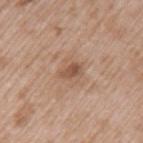Clinical impression: Recorded during total-body skin imaging; not selected for excision or biopsy. Context: A male patient, aged 48 to 52. The recorded lesion diameter is about 2.5 mm. Cropped from a whole-body photographic skin survey; the tile spans about 15 mm. The lesion is on the left upper arm.